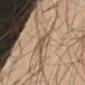| field | value |
|---|---|
| site | the right forearm |
| diameter | ~3.5 mm (longest diameter) |
| acquisition | 15 mm crop, total-body photography |
| patient | male, aged 63–67 |
| illumination | cross-polarized |
| TBP lesion metrics | an average lesion color of about L≈43 a*≈12 b*≈24 (CIELAB), a lesion–skin lightness drop of about 7, and a normalized lesion–skin contrast near 5.5; border irregularity of about 3 on a 0–10 scale and internal color variation of about 5 on a 0–10 scale |